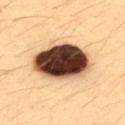Impression: Captured during whole-body skin photography for melanoma surveillance; the lesion was not biopsied. Background: A 15 mm close-up tile from a total-body photography series done for melanoma screening. An algorithmic analysis of the crop reported a footprint of about 28 mm². About 7 mm across. The lesion is located on the back. A female subject, roughly 35 years of age. The tile uses cross-polarized illumination.